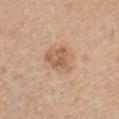No biopsy was performed on this lesion — it was imaged during a full skin examination and was not determined to be concerning. The lesion is on the chest. The patient is a female in their 40s. This is a white-light tile. A roughly 15 mm field-of-view crop from a total-body skin photograph.